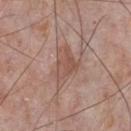Q: Was a biopsy performed?
A: imaged on a skin check; not biopsied
Q: What is the imaging modality?
A: ~15 mm crop, total-body skin-cancer survey
Q: What is the anatomic site?
A: the front of the torso
Q: How was the tile lit?
A: white-light illumination
Q: How large is the lesion?
A: about 5 mm
Q: Who is the patient?
A: male, in their mid- to late 70s